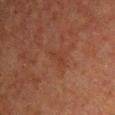Findings:
• biopsy status: no biopsy performed (imaged during a skin exam)
• location: the chest
• image-analysis metrics: a footprint of about 2 mm² and a symmetry-axis asymmetry near 0.4; an average lesion color of about L≈28 a*≈19 b*≈25 (CIELAB); a lesion-detection confidence of about 95/100
• illumination: cross-polarized
• diameter: ~2.5 mm (longest diameter)
• image source: ~15 mm crop, total-body skin-cancer survey
• subject: male, aged 58 to 62